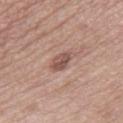The lesion was tiled from a total-body skin photograph and was not biopsied.
About 3 mm across.
A 15 mm close-up tile from a total-body photography series done for melanoma screening.
Captured under white-light illumination.
The patient is a female about 55 years old.
The lesion is located on the right thigh.